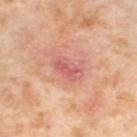workup: no biopsy performed (imaged during a skin exam); patient: female, in their mid-50s; tile lighting: cross-polarized; site: the left thigh; image: ~15 mm tile from a whole-body skin photo.